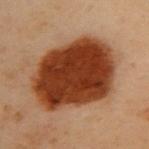{
  "biopsy_status": "not biopsied; imaged during a skin examination",
  "site": "upper back",
  "image": {
    "source": "total-body photography crop",
    "field_of_view_mm": 15
  },
  "patient": {
    "sex": "female",
    "age_approx": 60
  },
  "automated_metrics": {
    "cielab_L": 33,
    "cielab_a": 25,
    "cielab_b": 32,
    "vs_skin_darker_L": 19.0,
    "vs_skin_contrast_norm": 16.0
  },
  "lighting": "cross-polarized",
  "lesion_size": {
    "long_diameter_mm_approx": 9.5
  }
}